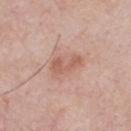Impression:
The lesion was tiled from a total-body skin photograph and was not biopsied.
Background:
Cropped from a total-body skin-imaging series; the visible field is about 15 mm. A male patient approximately 60 years of age. Measured at roughly 3.5 mm in maximum diameter. Located on the chest.